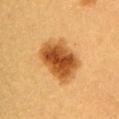Assessment:
The lesion was tiled from a total-body skin photograph and was not biopsied.
Acquisition and patient details:
The total-body-photography lesion software estimated about 16 CIELAB-L* units darker than the surrounding skin and a normalized border contrast of about 11. The lesion is located on the left upper arm. Captured under cross-polarized illumination. A female subject approximately 30 years of age. Longest diameter approximately 6 mm. A close-up tile cropped from a whole-body skin photograph, about 15 mm across.